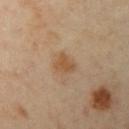follow-up: total-body-photography surveillance lesion; no biopsy
tile lighting: cross-polarized illumination
image: ~15 mm tile from a whole-body skin photo
patient: female, aged approximately 40
lesion diameter: about 2.5 mm
location: the left upper arm
image-analysis metrics: an average lesion color of about L≈54 a*≈17 b*≈35 (CIELAB) and a lesion–skin lightness drop of about 8; internal color variation of about 1.5 on a 0–10 scale and radial color variation of about 0.5